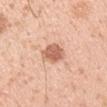Part of a total-body skin-imaging series; this lesion was reviewed on a skin check and was not flagged for biopsy. The tile uses white-light illumination. The recorded lesion diameter is about 3.5 mm. The subject is a male approximately 30 years of age. On the left upper arm. Cropped from a whole-body photographic skin survey; the tile spans about 15 mm.